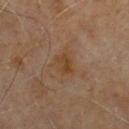Clinical impression: Captured during whole-body skin photography for melanoma surveillance; the lesion was not biopsied. Clinical summary: The lesion-visualizer software estimated a footprint of about 5.5 mm², an eccentricity of roughly 0.7, and a symmetry-axis asymmetry near 0.2. The software also gave an average lesion color of about L≈40 a*≈17 b*≈31 (CIELAB), a lesion–skin lightness drop of about 6, and a normalized lesion–skin contrast near 7. And it measured a border-irregularity index near 2.5/10 and peripheral color asymmetry of about 1. And it measured a classifier nevus-likeness of about 0/100 and a detector confidence of about 100 out of 100 that the crop contains a lesion. Measured at roughly 3 mm in maximum diameter. A male patient roughly 60 years of age. Cropped from a whole-body photographic skin survey; the tile spans about 15 mm. From the upper back.